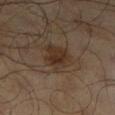Imaged during a routine full-body skin examination; the lesion was not biopsied and no histopathology is available. On the right lower leg. Cropped from a whole-body photographic skin survey; the tile spans about 15 mm. A male subject aged 63–67. The tile uses cross-polarized illumination.